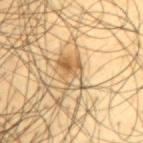The lesion was tiled from a total-body skin photograph and was not biopsied.
Longest diameter approximately 4.5 mm.
Located on the upper back.
Captured under cross-polarized illumination.
A male patient in their mid- to late 40s.
The total-body-photography lesion software estimated a footprint of about 8.5 mm², an outline eccentricity of about 0.8 (0 = round, 1 = elongated), and a shape-asymmetry score of about 0.35 (0 = symmetric). The analysis additionally found a border-irregularity index near 4/10, a within-lesion color-variation index near 10/10, and a peripheral color-asymmetry measure near 5.
A lesion tile, about 15 mm wide, cut from a 3D total-body photograph.